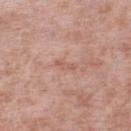Q: Is there a histopathology result?
A: no biopsy performed (imaged during a skin exam)
Q: Lesion location?
A: the left lower leg
Q: Who is the patient?
A: male, aged approximately 55
Q: How large is the lesion?
A: about 2.5 mm
Q: How was this image acquired?
A: total-body-photography crop, ~15 mm field of view